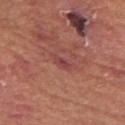The subject is a male approximately 80 years of age. Captured under white-light illumination. A 15 mm crop from a total-body photograph taken for skin-cancer surveillance. The lesion is on the arm. The total-body-photography lesion software estimated an average lesion color of about L≈44 a*≈29 b*≈23 (CIELAB), roughly 7 lightness units darker than nearby skin, and a lesion-to-skin contrast of about 6.5 (normalized; higher = more distinct). The software also gave a color-variation rating of about 2/10. And it measured an automated nevus-likeness rating near 0 out of 100 and a lesion-detection confidence of about 95/100.